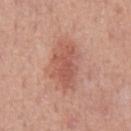workup: imaged on a skin check; not biopsied
image-analysis metrics: a shape eccentricity near 0.8; a lesion color around L≈56 a*≈25 b*≈29 in CIELAB and about 9 CIELAB-L* units darker than the surrounding skin; a border-irregularity rating of about 3.5/10, internal color variation of about 3 on a 0–10 scale, and peripheral color asymmetry of about 1
anatomic site: the chest
illumination: white-light
acquisition: ~15 mm crop, total-body skin-cancer survey
patient: male, aged 48 to 52
lesion diameter: ≈5 mm